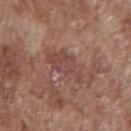Case summary:
• notes · catalogued during a skin exam; not biopsied
• automated metrics · a border-irregularity rating of about 4.5/10, internal color variation of about 2.5 on a 0–10 scale, and a peripheral color-asymmetry measure near 1
• patient · male, approximately 75 years of age
• tile lighting · white-light
• size · about 5 mm
• site · the chest
• image source · ~15 mm crop, total-body skin-cancer survey